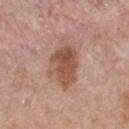workup = total-body-photography surveillance lesion; no biopsy | site = the back | tile lighting = white-light | subject = male, about 55 years old | acquisition = total-body-photography crop, ~15 mm field of view | size = ~5.5 mm (longest diameter).